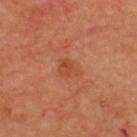The lesion was photographed on a routine skin check and not biopsied; there is no pathology result. On the back. A roughly 15 mm field-of-view crop from a total-body skin photograph. Captured under cross-polarized illumination. Approximately 2.5 mm at its widest. A male subject aged 63–67.